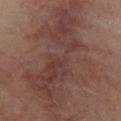{
  "automated_metrics": {
    "eccentricity": 0.9,
    "shape_asymmetry": 0.45,
    "cielab_L": 37,
    "cielab_a": 18,
    "cielab_b": 20,
    "vs_skin_darker_L": 8.0,
    "peripheral_color_asymmetry": 1.5
  },
  "lighting": "cross-polarized",
  "image": {
    "source": "total-body photography crop",
    "field_of_view_mm": 15
  },
  "patient": {
    "sex": "male",
    "age_approx": 65
  },
  "site": "leg",
  "lesion_size": {
    "long_diameter_mm_approx": 16.0
  }
}